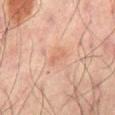Q: Was this lesion biopsied?
A: imaged on a skin check; not biopsied
Q: Who is the patient?
A: male, in their mid- to late 60s
Q: What kind of image is this?
A: ~15 mm tile from a whole-body skin photo
Q: Automated lesion metrics?
A: a lesion color around L≈50 a*≈20 b*≈27 in CIELAB, about 5 CIELAB-L* units darker than the surrounding skin, and a lesion-to-skin contrast of about 4.5 (normalized; higher = more distinct); a border-irregularity rating of about 5.5/10 and a within-lesion color-variation index near 0/10
Q: Lesion size?
A: ≈2.5 mm
Q: Lesion location?
A: the back